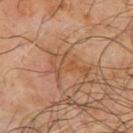| field | value |
|---|---|
| biopsy status | total-body-photography surveillance lesion; no biopsy |
| site | the upper back |
| lighting | cross-polarized |
| TBP lesion metrics | a footprint of about 8.5 mm², an outline eccentricity of about 0.8 (0 = round, 1 = elongated), and a symmetry-axis asymmetry near 0.65; a mean CIELAB color near L≈48 a*≈20 b*≈33, about 6 CIELAB-L* units darker than the surrounding skin, and a lesion-to-skin contrast of about 5 (normalized; higher = more distinct); a border-irregularity rating of about 9.5/10, internal color variation of about 3.5 on a 0–10 scale, and peripheral color asymmetry of about 1; a nevus-likeness score of about 0/100 and a detector confidence of about 75 out of 100 that the crop contains a lesion |
| diameter | about 4.5 mm |
| image | ~15 mm crop, total-body skin-cancer survey |
| subject | male, aged 63–67 |